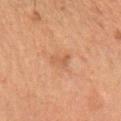{"biopsy_status": "not biopsied; imaged during a skin examination", "automated_metrics": {"cielab_L": 47, "cielab_a": 19, "cielab_b": 30, "vs_skin_darker_L": 6.0, "vs_skin_contrast_norm": 4.5, "nevus_likeness_0_100": 0}, "image": {"source": "total-body photography crop", "field_of_view_mm": 15}, "patient": {"sex": "female", "age_approx": 60}, "lighting": "cross-polarized", "lesion_size": {"long_diameter_mm_approx": 3.0}, "site": "left forearm"}